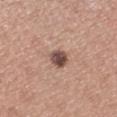Assessment:
Part of a total-body skin-imaging series; this lesion was reviewed on a skin check and was not flagged for biopsy.
Context:
A lesion tile, about 15 mm wide, cut from a 3D total-body photograph. The lesion is on the right lower leg. The total-body-photography lesion software estimated border irregularity of about 2 on a 0–10 scale, internal color variation of about 4.5 on a 0–10 scale, and radial color variation of about 1.5. The patient is a male about 60 years old.